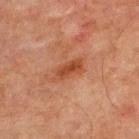Captured under cross-polarized illumination. From the chest. A male subject aged approximately 65. A lesion tile, about 15 mm wide, cut from a 3D total-body photograph.